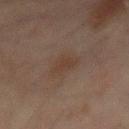A 15 mm close-up extracted from a 3D total-body photography capture. An algorithmic analysis of the crop reported an area of roughly 5.5 mm², a shape eccentricity near 0.65, and a symmetry-axis asymmetry near 0.35. The analysis additionally found a lesion-detection confidence of about 100/100. A male subject, in their mid-40s. Approximately 3 mm at its widest. The lesion is located on the mid back.